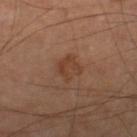Case summary:
* biopsy status · total-body-photography surveillance lesion; no biopsy
* subject · male, aged around 65
* location · the leg
* image source · 15 mm crop, total-body photography